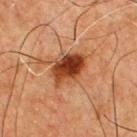No biopsy was performed on this lesion — it was imaged during a full skin examination and was not determined to be concerning. The subject is a male aged around 65. The tile uses cross-polarized illumination. A 15 mm crop from a total-body photograph taken for skin-cancer surveillance. The lesion is located on the chest. Automated image analysis of the tile measured an average lesion color of about L≈37 a*≈25 b*≈33 (CIELAB), roughly 15 lightness units darker than nearby skin, and a normalized border contrast of about 12. It also reported a border-irregularity rating of about 2/10 and internal color variation of about 7.5 on a 0–10 scale. Longest diameter approximately 5 mm.